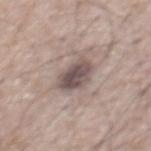<tbp_lesion>
<biopsy_status>not biopsied; imaged during a skin examination</biopsy_status>
<automated_metrics>
  <border_irregularity_0_10>2.0</border_irregularity_0_10>
  <color_variation_0_10>5.0</color_variation_0_10>
  <peripheral_color_asymmetry>2.0</peripheral_color_asymmetry>
  <lesion_detection_confidence_0_100>80</lesion_detection_confidence_0_100>
</automated_metrics>
<patient>
  <sex>male</sex>
  <age_approx>65</age_approx>
</patient>
<site>chest</site>
<image>
  <source>total-body photography crop</source>
  <field_of_view_mm>15</field_of_view_mm>
</image>
</tbp_lesion>